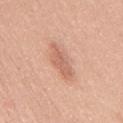Captured during whole-body skin photography for melanoma surveillance; the lesion was not biopsied. A roughly 15 mm field-of-view crop from a total-body skin photograph. The lesion is located on the mid back. A female patient, roughly 40 years of age.